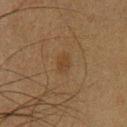Notes:
* notes · imaged on a skin check; not biopsied
* tile lighting · cross-polarized illumination
* lesion diameter · ~2.5 mm (longest diameter)
* image · total-body-photography crop, ~15 mm field of view
* patient · male, roughly 35 years of age
* anatomic site · the leg